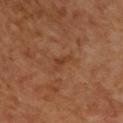biopsy status = imaged on a skin check; not biopsied | lesion size = ~3 mm (longest diameter) | body site = the chest | image source = ~15 mm crop, total-body skin-cancer survey | subject = male, in their 50s | lighting = cross-polarized.